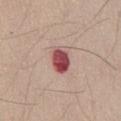biopsy status=total-body-photography surveillance lesion; no biopsy | body site=the abdomen | patient=male, in their mid- to late 40s | image source=~15 mm tile from a whole-body skin photo | tile lighting=white-light.